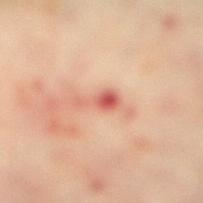This lesion was catalogued during total-body skin photography and was not selected for biopsy. Imaged with cross-polarized lighting. Automated tile analysis of the lesion measured a footprint of about 4 mm² and two-axis asymmetry of about 0.55. The software also gave an average lesion color of about L≈64 a*≈31 b*≈32 (CIELAB), about 13 CIELAB-L* units darker than the surrounding skin, and a lesion-to-skin contrast of about 7.5 (normalized; higher = more distinct). It also reported a border-irregularity index near 6/10 and internal color variation of about 4 on a 0–10 scale. It also reported an automated nevus-likeness rating near 0 out of 100 and a lesion-detection confidence of about 100/100. A female subject, in their mid-60s. Measured at roughly 3.5 mm in maximum diameter. From the right lower leg. A lesion tile, about 15 mm wide, cut from a 3D total-body photograph.